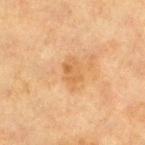• follow-up · total-body-photography surveillance lesion; no biopsy
• image · 15 mm crop, total-body photography
• diameter · ~3.5 mm (longest diameter)
• location · the left lower leg
• patient · female, aged approximately 40